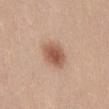imaging modality — ~15 mm crop, total-body skin-cancer survey
location — the front of the torso
size — about 4 mm
lighting — white-light
image-analysis metrics — a lesion color around L≈56 a*≈22 b*≈30 in CIELAB and roughly 13 lightness units darker than nearby skin; border irregularity of about 1.5 on a 0–10 scale
patient — female, aged 18–22